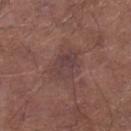This lesion was catalogued during total-body skin photography and was not selected for biopsy. Longest diameter approximately 5 mm. The subject is a male aged approximately 55. Captured under white-light illumination. The lesion is on the leg. This image is a 15 mm lesion crop taken from a total-body photograph.